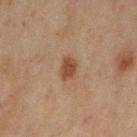On the left upper arm.
The lesion-visualizer software estimated an outline eccentricity of about 0.75 (0 = round, 1 = elongated) and a shape-asymmetry score of about 0.25 (0 = symmetric). The software also gave a mean CIELAB color near L≈41 a*≈18 b*≈29 and roughly 10 lightness units darker than nearby skin. The analysis additionally found border irregularity of about 2 on a 0–10 scale, a within-lesion color-variation index near 1/10, and a peripheral color-asymmetry measure near 0.5.
The recorded lesion diameter is about 3 mm.
A female patient aged 58–62.
This image is a 15 mm lesion crop taken from a total-body photograph.